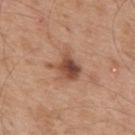Case summary:
• follow-up — catalogued during a skin exam; not biopsied
• illumination — white-light
• body site — the upper back
• acquisition — 15 mm crop, total-body photography
• subject — male, in their mid-50s